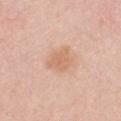<case>
  <biopsy_status>not biopsied; imaged during a skin examination</biopsy_status>
  <automated_metrics>
    <nevus_likeness_0_100>0</nevus_likeness_0_100>
    <lesion_detection_confidence_0_100>100</lesion_detection_confidence_0_100>
  </automated_metrics>
  <lighting>white-light</lighting>
  <image>
    <source>total-body photography crop</source>
    <field_of_view_mm>15</field_of_view_mm>
  </image>
  <site>chest</site>
  <patient>
    <sex>female</sex>
    <age_approx>30</age_approx>
  </patient>
  <lesion_size>
    <long_diameter_mm_approx>3.5</long_diameter_mm_approx>
  </lesion_size>
</case>